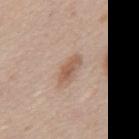Q: Is there a histopathology result?
A: total-body-photography surveillance lesion; no biopsy
Q: Patient demographics?
A: male, aged approximately 45
Q: What kind of image is this?
A: total-body-photography crop, ~15 mm field of view
Q: Where on the body is the lesion?
A: the mid back
Q: How large is the lesion?
A: ~4 mm (longest diameter)